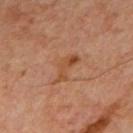Clinical impression: The lesion was photographed on a routine skin check and not biopsied; there is no pathology result. Clinical summary: An algorithmic analysis of the crop reported a lesion color around L≈48 a*≈23 b*≈35 in CIELAB, about 7 CIELAB-L* units darker than the surrounding skin, and a normalized border contrast of about 6.5. The analysis additionally found a border-irregularity rating of about 6/10 and internal color variation of about 4 on a 0–10 scale. Captured under cross-polarized illumination. Located on the mid back. About 4 mm across. A patient about 65 years old. A roughly 15 mm field-of-view crop from a total-body skin photograph.